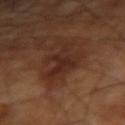workup: imaged on a skin check; not biopsied | anatomic site: the right upper arm | image: total-body-photography crop, ~15 mm field of view | subject: aged around 65 | lighting: cross-polarized | lesion diameter: about 5 mm.